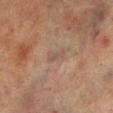notes = total-body-photography surveillance lesion; no biopsy
site = the right lower leg
illumination = cross-polarized
patient = male, aged 68 to 72
image = 15 mm crop, total-body photography
size = about 3 mm